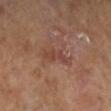Part of a total-body skin-imaging series; this lesion was reviewed on a skin check and was not flagged for biopsy. A male subject about 65 years old. Longest diameter approximately 4 mm. This image is a 15 mm lesion crop taken from a total-body photograph.